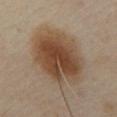follow-up=catalogued during a skin exam; not biopsied | patient=male, roughly 55 years of age | body site=the chest | acquisition=15 mm crop, total-body photography | illumination=cross-polarized illumination | lesion size=~7.5 mm (longest diameter).